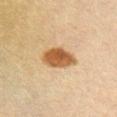{"biopsy_status": "not biopsied; imaged during a skin examination", "lighting": "cross-polarized", "patient": {"sex": "male", "age_approx": 60}, "site": "front of the torso", "lesion_size": {"long_diameter_mm_approx": 5.0}, "automated_metrics": {"area_mm2_approx": 9.5, "vs_skin_darker_L": 13.0, "vs_skin_contrast_norm": 10.5, "border_irregularity_0_10": 2.0, "color_variation_0_10": 3.5}, "image": {"source": "total-body photography crop", "field_of_view_mm": 15}}